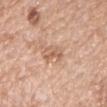Clinical impression:
Part of a total-body skin-imaging series; this lesion was reviewed on a skin check and was not flagged for biopsy.
Context:
On the arm. The patient is a female aged around 60. A 15 mm close-up tile from a total-body photography series done for melanoma screening.